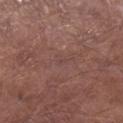Context:
This image is a 15 mm lesion crop taken from a total-body photograph. Longest diameter approximately 1 mm. The patient is a male about 55 years old. This is a white-light tile. Located on the right lower leg.
Diagnosis:
Histopathological examination showed a superficial basal cell carcinoma — a malignant lesion.